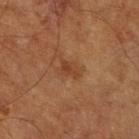Clinical impression: Captured during whole-body skin photography for melanoma surveillance; the lesion was not biopsied.